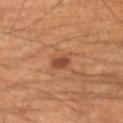lighting: cross-polarized
site: left forearm
lesion_size:
  long_diameter_mm_approx: 3.0
image:
  source: total-body photography crop
  field_of_view_mm: 15
automated_metrics:
  cielab_L: 46
  cielab_a: 23
  cielab_b: 33
  vs_skin_contrast_norm: 6.5
  nevus_likeness_0_100: 80
patient:
  sex: male
  age_approx: 50